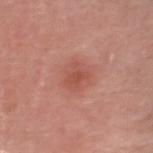workup = imaged on a skin check; not biopsied
subject = male, aged 58–62
image source = ~15 mm crop, total-body skin-cancer survey
body site = the head or neck
diameter = ~2.5 mm (longest diameter)
lighting = white-light illumination
automated lesion analysis = a peripheral color-asymmetry measure near 0.5